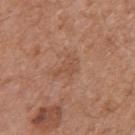Q: Was this lesion biopsied?
A: no biopsy performed (imaged during a skin exam)
Q: What did automated image analysis measure?
A: an average lesion color of about L≈51 a*≈22 b*≈31 (CIELAB), about 6 CIELAB-L* units darker than the surrounding skin, and a lesion-to-skin contrast of about 4.5 (normalized; higher = more distinct); an automated nevus-likeness rating near 0 out of 100 and lesion-presence confidence of about 100/100
Q: What is the anatomic site?
A: the arm
Q: What kind of image is this?
A: ~15 mm crop, total-body skin-cancer survey
Q: What are the patient's age and sex?
A: male, roughly 65 years of age
Q: How was the tile lit?
A: white-light
Q: How large is the lesion?
A: ≈3.5 mm